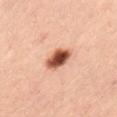biopsy_status: not biopsied; imaged during a skin examination
image:
  source: total-body photography crop
  field_of_view_mm: 15
patient:
  sex: female
  age_approx: 45
lesion_size:
  long_diameter_mm_approx: 3.5
lighting: cross-polarized
site: left thigh